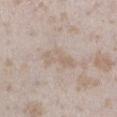• follow-up · no biopsy performed (imaged during a skin exam)
• subject · female, aged 23–27
• imaging modality · ~15 mm tile from a whole-body skin photo
• lesion diameter · ≈4.5 mm
• image-analysis metrics · a mean CIELAB color near L≈63 a*≈12 b*≈25, roughly 6 lightness units darker than nearby skin, and a normalized border contrast of about 5; a classifier nevus-likeness of about 0/100 and lesion-presence confidence of about 85/100
• site · the left lower leg
• tile lighting · white-light illumination